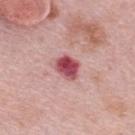Recorded during total-body skin imaging; not selected for excision or biopsy.
The lesion-visualizer software estimated an area of roughly 6 mm², an outline eccentricity of about 0.6 (0 = round, 1 = elongated), and two-axis asymmetry of about 0.25.
About 3 mm across.
A 15 mm close-up extracted from a 3D total-body photography capture.
A female subject, about 65 years old.
The lesion is on the upper back.
Imaged with white-light lighting.